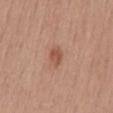Acquisition and patient details: A roughly 15 mm field-of-view crop from a total-body skin photograph. From the mid back. The lesion-visualizer software estimated an area of roughly 4 mm², an outline eccentricity of about 0.7 (0 = round, 1 = elongated), and two-axis asymmetry of about 0.2. It also reported a lesion color around L≈53 a*≈24 b*≈30 in CIELAB, a lesion–skin lightness drop of about 9, and a normalized border contrast of about 6.5. This is a white-light tile. The subject is a female aged 53–57. Measured at roughly 2.5 mm in maximum diameter.